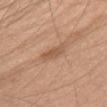{"patient": {"sex": "female", "age_approx": 25}, "automated_metrics": {"cielab_L": 55, "cielab_a": 20, "cielab_b": 33, "vs_skin_contrast_norm": 6.0, "border_irregularity_0_10": 3.0, "color_variation_0_10": 2.0, "peripheral_color_asymmetry": 0.5, "nevus_likeness_0_100": 0, "lesion_detection_confidence_0_100": 95}, "site": "chest", "image": {"source": "total-body photography crop", "field_of_view_mm": 15}, "lesion_size": {"long_diameter_mm_approx": 3.5}, "lighting": "white-light"}